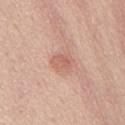Q: Where on the body is the lesion?
A: the chest
Q: Patient demographics?
A: female, approximately 45 years of age
Q: How was this image acquired?
A: ~15 mm tile from a whole-body skin photo